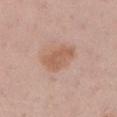No biopsy was performed on this lesion — it was imaged during a full skin examination and was not determined to be concerning. Located on the right lower leg. The subject is a female aged 28 to 32. Approximately 4 mm at its widest. Imaged with white-light lighting. A roughly 15 mm field-of-view crop from a total-body skin photograph. The total-body-photography lesion software estimated a lesion color around L≈59 a*≈20 b*≈30 in CIELAB. The analysis additionally found a nevus-likeness score of about 25/100 and lesion-presence confidence of about 100/100.